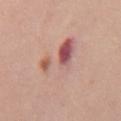Notes:
- follow-up: no biopsy performed (imaged during a skin exam)
- image: 15 mm crop, total-body photography
- TBP lesion metrics: a lesion color around L≈60 a*≈22 b*≈24 in CIELAB and a lesion-to-skin contrast of about 6 (normalized; higher = more distinct); a border-irregularity index near 5.5/10, a within-lesion color-variation index near 10/10, and peripheral color asymmetry of about 5.5; a classifier nevus-likeness of about 5/100 and a detector confidence of about 100 out of 100 that the crop contains a lesion
- lesion diameter: ~6.5 mm (longest diameter)
- anatomic site: the mid back
- patient: male, approximately 55 years of age
- tile lighting: white-light illumination